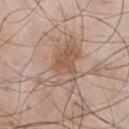Case summary:
* notes · total-body-photography surveillance lesion; no biopsy
* site · the chest
* image · ~15 mm crop, total-body skin-cancer survey
* automated lesion analysis · a footprint of about 16 mm², an outline eccentricity of about 0.8 (0 = round, 1 = elongated), and a symmetry-axis asymmetry near 0.35; a mean CIELAB color near L≈57 a*≈16 b*≈28, about 9 CIELAB-L* units darker than the surrounding skin, and a lesion-to-skin contrast of about 6.5 (normalized; higher = more distinct); a nevus-likeness score of about 20/100 and a lesion-detection confidence of about 100/100
* subject · male, aged around 55
* lighting · white-light
* size · about 6.5 mm